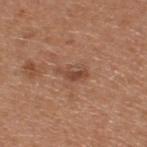Findings:
- biopsy status · catalogued during a skin exam; not biopsied
- site · the upper back
- automated metrics · an automated nevus-likeness rating near 5 out of 100 and a lesion-detection confidence of about 100/100
- tile lighting · white-light
- imaging modality · total-body-photography crop, ~15 mm field of view
- lesion size · ~3.5 mm (longest diameter)
- patient · male, roughly 65 years of age